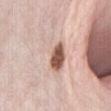This lesion was catalogued during total-body skin photography and was not selected for biopsy. Longest diameter approximately 5.5 mm. The patient is a female about 60 years old. Automated tile analysis of the lesion measured an outline eccentricity of about 0.8 (0 = round, 1 = elongated) and two-axis asymmetry of about 0.35. The analysis additionally found a lesion color around L≈64 a*≈18 b*≈25 in CIELAB, roughly 12 lightness units darker than nearby skin, and a normalized border contrast of about 7.5. The lesion is on the abdomen. The tile uses white-light illumination. A region of skin cropped from a whole-body photographic capture, roughly 15 mm wide.